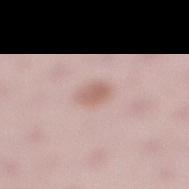Imaged during a routine full-body skin examination; the lesion was not biopsied and no histopathology is available.
A 15 mm crop from a total-body photograph taken for skin-cancer surveillance.
The lesion is located on the right lower leg.
A female subject in their 30s.